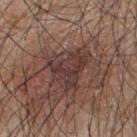Findings:
– subject: male, approximately 45 years of age
– tile lighting: white-light
– image: 15 mm crop, total-body photography
– site: the upper back
– lesion size: ~5 mm (longest diameter)
– TBP lesion metrics: a lesion area of about 14 mm² and a shape eccentricity near 0.55; an average lesion color of about L≈37 a*≈18 b*≈21 (CIELAB), roughly 8 lightness units darker than nearby skin, and a normalized lesion–skin contrast near 7; a border-irregularity index near 6/10, internal color variation of about 4 on a 0–10 scale, and peripheral color asymmetry of about 1.5; a classifier nevus-likeness of about 5/100 and a detector confidence of about 65 out of 100 that the crop contains a lesion